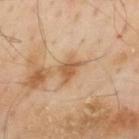| field | value |
|---|---|
| workup | imaged on a skin check; not biopsied |
| TBP lesion metrics | a border-irregularity index near 3/10, a color-variation rating of about 2/10, and peripheral color asymmetry of about 1; a nevus-likeness score of about 0/100 |
| subject | male, in their mid- to late 50s |
| body site | the mid back |
| image | total-body-photography crop, ~15 mm field of view |